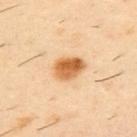Assessment:
No biopsy was performed on this lesion — it was imaged during a full skin examination and was not determined to be concerning.
Clinical summary:
A 15 mm close-up extracted from a 3D total-body photography capture. The patient is a male aged approximately 40. The lesion is on the upper back. Longest diameter approximately 4 mm.